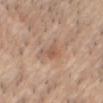workup = catalogued during a skin exam; not biopsied
image-analysis metrics = a lesion color around L≈56 a*≈19 b*≈30 in CIELAB, about 8 CIELAB-L* units darker than the surrounding skin, and a normalized lesion–skin contrast near 5.5; a nevus-likeness score of about 0/100
subject = male, approximately 35 years of age
location = the head or neck
lesion size = ≈2.5 mm
acquisition = ~15 mm tile from a whole-body skin photo
tile lighting = white-light illumination